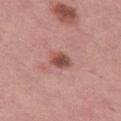Clinical impression: Captured during whole-body skin photography for melanoma surveillance; the lesion was not biopsied. Background: The patient is a female aged 48–52. Automated image analysis of the tile measured a lesion area of about 4.5 mm², an outline eccentricity of about 0.65 (0 = round, 1 = elongated), and a shape-asymmetry score of about 0.15 (0 = symmetric). And it measured border irregularity of about 1.5 on a 0–10 scale and internal color variation of about 4 on a 0–10 scale. And it measured a nevus-likeness score of about 80/100. About 3 mm across. A 15 mm close-up extracted from a 3D total-body photography capture. Captured under white-light illumination. The lesion is located on the leg.